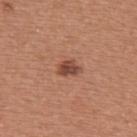notes: total-body-photography surveillance lesion; no biopsy | image: ~15 mm tile from a whole-body skin photo | subject: female, aged around 50 | anatomic site: the upper back | automated metrics: a lesion area of about 4 mm², an outline eccentricity of about 0.7 (0 = round, 1 = elongated), and a symmetry-axis asymmetry near 0.25; a mean CIELAB color near L≈46 a*≈24 b*≈29 and a normalized lesion–skin contrast near 9; a color-variation rating of about 3/10 and peripheral color asymmetry of about 1; a classifier nevus-likeness of about 85/100 and lesion-presence confidence of about 100/100.